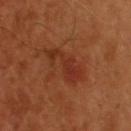Q: Was this lesion biopsied?
A: total-body-photography surveillance lesion; no biopsy
Q: Patient demographics?
A: male, in their mid-50s
Q: What is the lesion's diameter?
A: about 6 mm
Q: What is the imaging modality?
A: ~15 mm crop, total-body skin-cancer survey
Q: How was the tile lit?
A: cross-polarized illumination
Q: Automated lesion metrics?
A: a lesion color around L≈32 a*≈27 b*≈31 in CIELAB and roughly 7 lightness units darker than nearby skin; a nevus-likeness score of about 0/100 and a lesion-detection confidence of about 100/100
Q: Lesion location?
A: the upper back